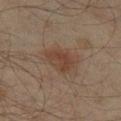This lesion was catalogued during total-body skin photography and was not selected for biopsy. Longest diameter approximately 4 mm. Imaged with cross-polarized lighting. The lesion is located on the left thigh. The patient is a male aged 58 to 62. The lesion-visualizer software estimated an eccentricity of roughly 0.75 and a shape-asymmetry score of about 0.25 (0 = symmetric). The software also gave a mean CIELAB color near L≈37 a*≈16 b*≈24, about 7 CIELAB-L* units darker than the surrounding skin, and a normalized border contrast of about 6.5. The analysis additionally found border irregularity of about 2.5 on a 0–10 scale, a within-lesion color-variation index near 2.5/10, and radial color variation of about 1. A region of skin cropped from a whole-body photographic capture, roughly 15 mm wide.